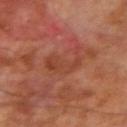Findings:
- notes: no biopsy performed (imaged during a skin exam)
- subject: male, aged approximately 70
- image source: ~15 mm tile from a whole-body skin photo
- anatomic site: the right upper arm
- diameter: ≈4 mm
- automated metrics: an area of roughly 9 mm², an eccentricity of roughly 0.65, and a shape-asymmetry score of about 0.5 (0 = symmetric); a normalized lesion–skin contrast near 5
- lighting: cross-polarized illumination